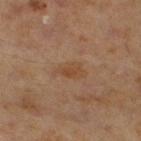Imaged during a routine full-body skin examination; the lesion was not biopsied and no histopathology is available.
An algorithmic analysis of the crop reported an area of roughly 5.5 mm² and two-axis asymmetry of about 0.4. The software also gave roughly 5 lightness units darker than nearby skin and a normalized border contrast of about 6. And it measured border irregularity of about 4 on a 0–10 scale, a within-lesion color-variation index near 2/10, and a peripheral color-asymmetry measure near 0.5.
About 3.5 mm across.
A close-up tile cropped from a whole-body skin photograph, about 15 mm across.
The subject is a male aged 58 to 62.
Captured under cross-polarized illumination.
Located on the left lower leg.